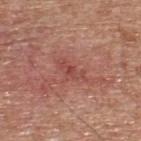Notes:
• follow-up: no biopsy performed (imaged during a skin exam)
• subject: male, aged approximately 65
• body site: the upper back
• image: ~15 mm crop, total-body skin-cancer survey